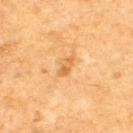This lesion was catalogued during total-body skin photography and was not selected for biopsy. A male patient in their 50s. The lesion-visualizer software estimated a lesion area of about 3 mm², an eccentricity of roughly 0.9, and a shape-asymmetry score of about 0.25 (0 = symmetric). It also reported about 8 CIELAB-L* units darker than the surrounding skin and a normalized lesion–skin contrast near 6. And it measured a color-variation rating of about 0/10 and radial color variation of about 0. The analysis additionally found a nevus-likeness score of about 10/100. Approximately 3 mm at its widest. From the upper back. A 15 mm crop from a total-body photograph taken for skin-cancer surveillance. This is a cross-polarized tile.